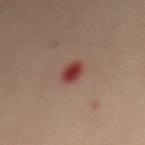Q: Lesion size?
A: ~3 mm (longest diameter)
Q: What lighting was used for the tile?
A: cross-polarized
Q: Who is the patient?
A: female, aged around 45
Q: How was this image acquired?
A: ~15 mm crop, total-body skin-cancer survey
Q: Where on the body is the lesion?
A: the mid back